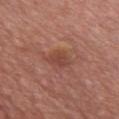{"biopsy_status": "not biopsied; imaged during a skin examination", "lesion_size": {"long_diameter_mm_approx": 3.0}, "patient": {"sex": "male", "age_approx": 65}, "lighting": "white-light", "site": "chest", "image": {"source": "total-body photography crop", "field_of_view_mm": 15}}